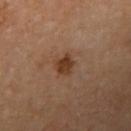Part of a total-body skin-imaging series; this lesion was reviewed on a skin check and was not flagged for biopsy. An algorithmic analysis of the crop reported a lesion area of about 4 mm², a shape eccentricity near 0.65, and two-axis asymmetry of about 0.3. The analysis additionally found a mean CIELAB color near L≈37 a*≈21 b*≈31, roughly 10 lightness units darker than nearby skin, and a normalized border contrast of about 9.5. The analysis additionally found a border-irregularity index near 2.5/10, a color-variation rating of about 2.5/10, and a peripheral color-asymmetry measure near 0.5. The analysis additionally found an automated nevus-likeness rating near 90 out of 100 and a lesion-detection confidence of about 100/100. The patient is a male aged 63–67. Cropped from a total-body skin-imaging series; the visible field is about 15 mm. About 2.5 mm across. On the right upper arm.